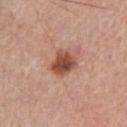Context: Cropped from a total-body skin-imaging series; the visible field is about 15 mm. A male patient in their mid-70s. On the chest. Measured at roughly 3.5 mm in maximum diameter. Imaged with white-light lighting.